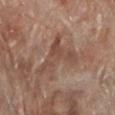Assessment:
Imaged during a routine full-body skin examination; the lesion was not biopsied and no histopathology is available.
Acquisition and patient details:
Imaged with white-light lighting. A male subject, aged 68 to 72. Longest diameter approximately 6.5 mm. Automated tile analysis of the lesion measured a footprint of about 12 mm², a shape eccentricity near 0.8, and a symmetry-axis asymmetry near 0.8. Located on the left lower leg. This image is a 15 mm lesion crop taken from a total-body photograph.